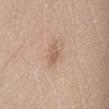Findings:
• workup · total-body-photography surveillance lesion; no biopsy
• location · the left lower leg
• illumination · white-light illumination
• lesion size · ~2.5 mm (longest diameter)
• subject · female, aged approximately 40
• acquisition · total-body-photography crop, ~15 mm field of view